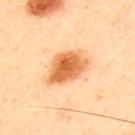{
  "biopsy_status": "not biopsied; imaged during a skin examination",
  "patient": {
    "sex": "male",
    "age_approx": 45
  },
  "site": "upper back",
  "lesion_size": {
    "long_diameter_mm_approx": 5.0
  },
  "image": {
    "source": "total-body photography crop",
    "field_of_view_mm": 15
  },
  "lighting": "cross-polarized"
}